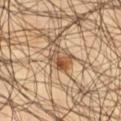| field | value |
|---|---|
| follow-up | catalogued during a skin exam; not biopsied |
| patient | male, approximately 45 years of age |
| tile lighting | cross-polarized illumination |
| imaging modality | total-body-photography crop, ~15 mm field of view |
| diameter | ~3 mm (longest diameter) |
| location | the leg |
| automated lesion analysis | an area of roughly 5 mm², an eccentricity of roughly 0.8, and a symmetry-axis asymmetry near 0.5; an average lesion color of about L≈50 a*≈19 b*≈34 (CIELAB), about 13 CIELAB-L* units darker than the surrounding skin, and a normalized border contrast of about 9.5; a classifier nevus-likeness of about 70/100 and lesion-presence confidence of about 100/100 |